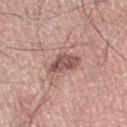Image and clinical context:
A male subject, roughly 70 years of age. Approximately 4 mm at its widest. An algorithmic analysis of the crop reported a footprint of about 7.5 mm², an outline eccentricity of about 0.8 (0 = round, 1 = elongated), and a shape-asymmetry score of about 0.25 (0 = symmetric). It also reported a border-irregularity rating of about 3/10 and radial color variation of about 1.5. It also reported a nevus-likeness score of about 40/100 and a lesion-detection confidence of about 100/100. A region of skin cropped from a whole-body photographic capture, roughly 15 mm wide. The lesion is on the right thigh.